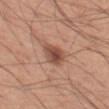workup = catalogued during a skin exam; not biopsied
image = ~15 mm crop, total-body skin-cancer survey
patient = male, aged around 55
anatomic site = the left thigh